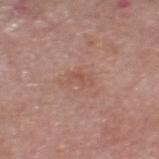Part of a total-body skin-imaging series; this lesion was reviewed on a skin check and was not flagged for biopsy. Captured under white-light illumination. A male subject, roughly 50 years of age. From the head or neck. A close-up tile cropped from a whole-body skin photograph, about 15 mm across. Approximately 2.5 mm at its widest. The total-body-photography lesion software estimated an area of roughly 4 mm², an outline eccentricity of about 0.75 (0 = round, 1 = elongated), and a symmetry-axis asymmetry near 0.35. And it measured an average lesion color of about L≈53 a*≈22 b*≈27 (CIELAB), about 6 CIELAB-L* units darker than the surrounding skin, and a lesion-to-skin contrast of about 5 (normalized; higher = more distinct). The analysis additionally found a nevus-likeness score of about 0/100 and lesion-presence confidence of about 100/100.